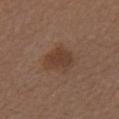biopsy_status: not biopsied; imaged during a skin examination
patient:
  sex: female
  age_approx: 40
image:
  source: total-body photography crop
  field_of_view_mm: 15
lesion_size:
  long_diameter_mm_approx: 4.0
site: right forearm